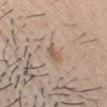The lesion was tiled from a total-body skin photograph and was not biopsied.
This is a white-light tile.
On the head or neck.
An algorithmic analysis of the crop reported an eccentricity of roughly 0.9 and a symmetry-axis asymmetry near 0.25. The software also gave a border-irregularity rating of about 2.5/10. And it measured a detector confidence of about 100 out of 100 that the crop contains a lesion.
Cropped from a whole-body photographic skin survey; the tile spans about 15 mm.
The lesion's longest dimension is about 2.5 mm.
A male subject aged 23 to 27.